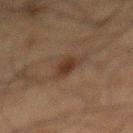| field | value |
|---|---|
| notes | no biopsy performed (imaged during a skin exam) |
| lighting | cross-polarized |
| location | the mid back |
| diameter | ≈3 mm |
| subject | male, about 60 years old |
| image | 15 mm crop, total-body photography |